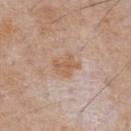Recorded during total-body skin imaging; not selected for excision or biopsy. Located on the chest. A male patient, aged 63–67. A close-up tile cropped from a whole-body skin photograph, about 15 mm across. The total-body-photography lesion software estimated a mean CIELAB color near L≈59 a*≈19 b*≈31 and a lesion-to-skin contrast of about 7 (normalized; higher = more distinct). And it measured border irregularity of about 4 on a 0–10 scale. The recorded lesion diameter is about 3 mm. The tile uses white-light illumination.